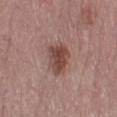Q: Was a biopsy performed?
A: catalogued during a skin exam; not biopsied
Q: What is the lesion's diameter?
A: ~4 mm (longest diameter)
Q: Where on the body is the lesion?
A: the right thigh
Q: How was this image acquired?
A: ~15 mm tile from a whole-body skin photo
Q: Patient demographics?
A: female, in their mid- to late 60s
Q: How was the tile lit?
A: white-light illumination